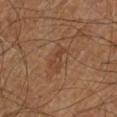Q: Was a biopsy performed?
A: imaged on a skin check; not biopsied
Q: What are the patient's age and sex?
A: male, approximately 60 years of age
Q: How was the tile lit?
A: cross-polarized illumination
Q: Lesion location?
A: the left lower leg
Q: How was this image acquired?
A: total-body-photography crop, ~15 mm field of view
Q: Lesion size?
A: ~2.5 mm (longest diameter)